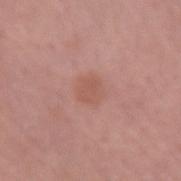  biopsy_status: not biopsied; imaged during a skin examination
  patient:
    sex: male
    age_approx: 60
  site: left forearm
  lighting: white-light
  lesion_size:
    long_diameter_mm_approx: 3.0
  automated_metrics:
    color_variation_0_10: 1.5
    peripheral_color_asymmetry: 0.5
  image:
    source: total-body photography crop
    field_of_view_mm: 15